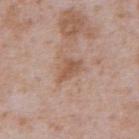The lesion was photographed on a routine skin check and not biopsied; there is no pathology result. Located on the abdomen. A male subject, roughly 65 years of age. A close-up tile cropped from a whole-body skin photograph, about 15 mm across. Imaged with white-light lighting. Automated image analysis of the tile measured an automated nevus-likeness rating near 0 out of 100 and lesion-presence confidence of about 100/100. Longest diameter approximately 3 mm.